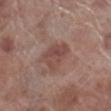Captured during whole-body skin photography for melanoma surveillance; the lesion was not biopsied.
A male patient aged approximately 70.
Located on the right lower leg.
This is a white-light tile.
About 4 mm across.
A region of skin cropped from a whole-body photographic capture, roughly 15 mm wide.